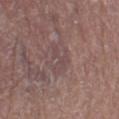Assessment:
Imaged during a routine full-body skin examination; the lesion was not biopsied and no histopathology is available.
Background:
A roughly 15 mm field-of-view crop from a total-body skin photograph. Captured under white-light illumination. The lesion is on the leg. A male patient, roughly 65 years of age. Automated image analysis of the tile measured a lesion color around L≈45 a*≈17 b*≈17 in CIELAB and a lesion–skin lightness drop of about 5. It also reported a nevus-likeness score of about 0/100 and lesion-presence confidence of about 65/100. About 2.5 mm across.